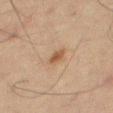biopsy status: imaged on a skin check; not biopsied | acquisition: ~15 mm tile from a whole-body skin photo | lesion size: ~2.5 mm (longest diameter) | patient: male, roughly 65 years of age | illumination: cross-polarized | location: the leg.